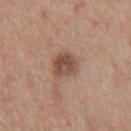Q: Is there a histopathology result?
A: no biopsy performed (imaged during a skin exam)
Q: Where on the body is the lesion?
A: the front of the torso
Q: How was this image acquired?
A: 15 mm crop, total-body photography
Q: Who is the patient?
A: male, approximately 50 years of age